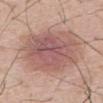No biopsy was performed on this lesion — it was imaged during a full skin examination and was not determined to be concerning.